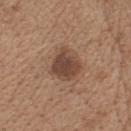Assessment:
This lesion was catalogued during total-body skin photography and was not selected for biopsy.
Image and clinical context:
Approximately 3.5 mm at its widest. The subject is a female about 70 years old. The lesion is located on the head or neck. Automated tile analysis of the lesion measured a lesion color around L≈44 a*≈18 b*≈27 in CIELAB and about 12 CIELAB-L* units darker than the surrounding skin. And it measured a border-irregularity rating of about 2.5/10, internal color variation of about 3 on a 0–10 scale, and radial color variation of about 1. A roughly 15 mm field-of-view crop from a total-body skin photograph. Imaged with white-light lighting.